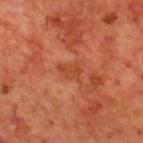{
  "image": {
    "source": "total-body photography crop",
    "field_of_view_mm": 15
  },
  "lighting": "cross-polarized",
  "site": "upper back",
  "automated_metrics": {
    "area_mm2_approx": 4.0,
    "eccentricity": 0.75,
    "shape_asymmetry": 0.45,
    "cielab_L": 45,
    "cielab_a": 30,
    "cielab_b": 38,
    "vs_skin_darker_L": 6.0,
    "vs_skin_contrast_norm": 5.5,
    "color_variation_0_10": 1.5,
    "peripheral_color_asymmetry": 0.5,
    "nevus_likeness_0_100": 0
  },
  "patient": {
    "sex": "male",
    "age_approx": 70
  }
}